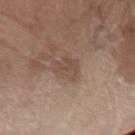Q: Is there a histopathology result?
A: catalogued during a skin exam; not biopsied
Q: How was the tile lit?
A: white-light
Q: What is the anatomic site?
A: the left forearm
Q: How large is the lesion?
A: ≈3.5 mm
Q: What kind of image is this?
A: ~15 mm crop, total-body skin-cancer survey
Q: Who is the patient?
A: male, roughly 70 years of age